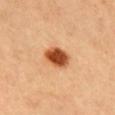Q: Was this lesion biopsied?
A: no biopsy performed (imaged during a skin exam)
Q: How was the tile lit?
A: cross-polarized illumination
Q: What kind of image is this?
A: ~15 mm crop, total-body skin-cancer survey
Q: What is the lesion's diameter?
A: ~3.5 mm (longest diameter)
Q: What are the patient's age and sex?
A: female, aged 33–37
Q: Where on the body is the lesion?
A: the head or neck